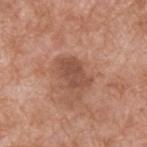notes — catalogued during a skin exam; not biopsied | patient — male, roughly 60 years of age | location — the upper back | image — ~15 mm tile from a whole-body skin photo | image-analysis metrics — a lesion area of about 9 mm², an outline eccentricity of about 0.8 (0 = round, 1 = elongated), and a shape-asymmetry score of about 0.3 (0 = symmetric); an average lesion color of about L≈50 a*≈22 b*≈29 (CIELAB), roughly 9 lightness units darker than nearby skin, and a lesion-to-skin contrast of about 6.5 (normalized; higher = more distinct).